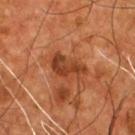Recorded during total-body skin imaging; not selected for excision or biopsy.
A region of skin cropped from a whole-body photographic capture, roughly 15 mm wide.
The lesion is located on the chest.
The lesion's longest dimension is about 4.5 mm.
Captured under cross-polarized illumination.
A male subject aged approximately 55.
An algorithmic analysis of the crop reported an average lesion color of about L≈38 a*≈26 b*≈34 (CIELAB), about 10 CIELAB-L* units darker than the surrounding skin, and a normalized lesion–skin contrast near 8.5. It also reported a color-variation rating of about 4/10 and peripheral color asymmetry of about 1. The software also gave an automated nevus-likeness rating near 0 out of 100.